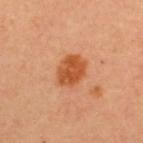Q: Was this lesion biopsied?
A: catalogued during a skin exam; not biopsied
Q: What did automated image analysis measure?
A: an outline eccentricity of about 0.65 (0 = round, 1 = elongated) and two-axis asymmetry of about 0.15; roughly 12 lightness units darker than nearby skin and a normalized lesion–skin contrast near 9
Q: Lesion location?
A: the upper back
Q: How was the tile lit?
A: cross-polarized
Q: How was this image acquired?
A: ~15 mm crop, total-body skin-cancer survey
Q: Patient demographics?
A: female, in their mid-40s
Q: What is the lesion's diameter?
A: ~4 mm (longest diameter)